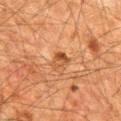biopsy status: total-body-photography surveillance lesion; no biopsy | patient: male, approximately 60 years of age | image source: ~15 mm tile from a whole-body skin photo | site: the mid back.